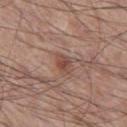Q: How large is the lesion?
A: ≈2.5 mm
Q: Automated lesion metrics?
A: a detector confidence of about 100 out of 100 that the crop contains a lesion
Q: How was this image acquired?
A: 15 mm crop, total-body photography
Q: Illumination type?
A: white-light
Q: Where on the body is the lesion?
A: the leg
Q: What are the patient's age and sex?
A: male, in their 60s